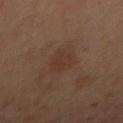Q: What kind of image is this?
A: total-body-photography crop, ~15 mm field of view
Q: Where on the body is the lesion?
A: the arm
Q: Patient demographics?
A: female, about 45 years old